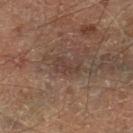| field | value |
|---|---|
| notes | total-body-photography surveillance lesion; no biopsy |
| patient | male, approximately 70 years of age |
| lesion diameter | about 3 mm |
| image source | ~15 mm tile from a whole-body skin photo |
| TBP lesion metrics | a lesion color around L≈38 a*≈17 b*≈22 in CIELAB and a lesion-to-skin contrast of about 5.5 (normalized; higher = more distinct); border irregularity of about 5.5 on a 0–10 scale, a color-variation rating of about 2.5/10, and peripheral color asymmetry of about 1; a nevus-likeness score of about 0/100 and a lesion-detection confidence of about 55/100 |
| tile lighting | cross-polarized illumination |
| body site | the left lower leg |